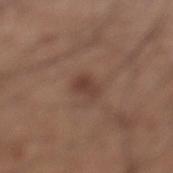On the leg.
This is a white-light tile.
The lesion's longest dimension is about 3 mm.
The patient is a male roughly 60 years of age.
A lesion tile, about 15 mm wide, cut from a 3D total-body photograph.